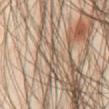Recorded during total-body skin imaging; not selected for excision or biopsy. Located on the abdomen. Automated image analysis of the tile measured a lesion area of about 2.5 mm², an eccentricity of roughly 0.95, and a symmetry-axis asymmetry near 0.4. It also reported roughly 5 lightness units darker than nearby skin and a lesion-to-skin contrast of about 4 (normalized; higher = more distinct). It also reported a color-variation rating of about 0/10 and a peripheral color-asymmetry measure near 0. Cropped from a total-body skin-imaging series; the visible field is about 15 mm. A male patient aged 43 to 47. The tile uses cross-polarized illumination. Measured at roughly 3.5 mm in maximum diameter.